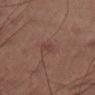follow-up: total-body-photography surveillance lesion; no biopsy
illumination: cross-polarized
patient: male, aged 58 to 62
body site: the right thigh
image: total-body-photography crop, ~15 mm field of view
diameter: ~2.5 mm (longest diameter)
image-analysis metrics: a footprint of about 3 mm², an outline eccentricity of about 0.75 (0 = round, 1 = elongated), and a shape-asymmetry score of about 0.3 (0 = symmetric); an average lesion color of about L≈35 a*≈18 b*≈19 (CIELAB), roughly 5 lightness units darker than nearby skin, and a lesion-to-skin contrast of about 5 (normalized; higher = more distinct); a nevus-likeness score of about 10/100 and a detector confidence of about 100 out of 100 that the crop contains a lesion